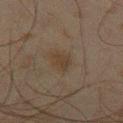Captured during whole-body skin photography for melanoma surveillance; the lesion was not biopsied. Automated tile analysis of the lesion measured a footprint of about 5 mm², an eccentricity of roughly 0.6, and two-axis asymmetry of about 0.25. The software also gave a lesion color around L≈31 a*≈10 b*≈22 in CIELAB and a normalized border contrast of about 6. It also reported a nevus-likeness score of about 65/100 and a lesion-detection confidence of about 100/100. This image is a 15 mm lesion crop taken from a total-body photograph. A male patient, aged 43–47. Located on the right thigh. The tile uses cross-polarized illumination. About 3 mm across.